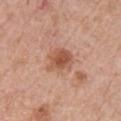  biopsy_status: not biopsied; imaged during a skin examination
  patient:
    sex: male
    age_approx: 65
  lesion_size:
    long_diameter_mm_approx: 3.5
  image:
    source: total-body photography crop
    field_of_view_mm: 15
  lighting: white-light
  site: front of the torso